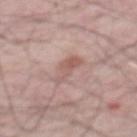Notes:
• follow-up — imaged on a skin check; not biopsied
• lighting — white-light illumination
• subject — male, approximately 65 years of age
• location — the mid back
• image source — ~15 mm tile from a whole-body skin photo
• lesion size — ~4.5 mm (longest diameter)